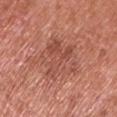Captured during whole-body skin photography for melanoma surveillance; the lesion was not biopsied.
Measured at roughly 6 mm in maximum diameter.
A male patient aged 63–67.
Cropped from a total-body skin-imaging series; the visible field is about 15 mm.
The lesion is located on the left upper arm.